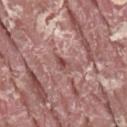<lesion>
<biopsy_status>not biopsied; imaged during a skin examination</biopsy_status>
<lesion_size>
  <long_diameter_mm_approx>2.5</long_diameter_mm_approx>
</lesion_size>
<site>right thigh</site>
<image>
  <source>total-body photography crop</source>
  <field_of_view_mm>15</field_of_view_mm>
</image>
<lighting>white-light</lighting>
<patient>
  <sex>male</sex>
  <age_approx>40</age_approx>
</patient>
</lesion>